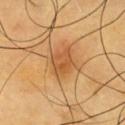This lesion was catalogued during total-body skin photography and was not selected for biopsy. A 15 mm close-up tile from a total-body photography series done for melanoma screening. The lesion is on the chest. A male patient aged around 60.